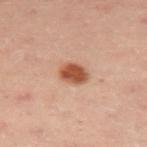biopsy status: no biopsy performed (imaged during a skin exam)
location: the upper back
patient: female, about 40 years old
tile lighting: cross-polarized illumination
lesion diameter: ≈3 mm
image: total-body-photography crop, ~15 mm field of view
automated lesion analysis: about 15 CIELAB-L* units darker than the surrounding skin and a normalized lesion–skin contrast near 10.5; internal color variation of about 3.5 on a 0–10 scale and radial color variation of about 1.5; a classifier nevus-likeness of about 100/100